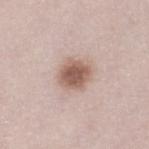Impression: Captured during whole-body skin photography for melanoma surveillance; the lesion was not biopsied. Background: Captured under white-light illumination. Approximately 3.5 mm at its widest. Located on the left lower leg. The patient is a female aged approximately 50. Cropped from a total-body skin-imaging series; the visible field is about 15 mm.